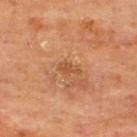{"biopsy_status": "not biopsied; imaged during a skin examination", "lesion_size": {"long_diameter_mm_approx": 2.5}, "image": {"source": "total-body photography crop", "field_of_view_mm": 15}, "patient": {"sex": "male", "age_approx": 65}, "site": "upper back", "automated_metrics": {"area_mm2_approx": 3.0, "eccentricity": 0.85, "border_irregularity_0_10": 3.5, "color_variation_0_10": 1.0, "nevus_likeness_0_100": 0, "lesion_detection_confidence_0_100": 100}, "lighting": "cross-polarized"}